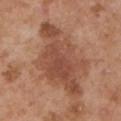Impression:
Imaged during a routine full-body skin examination; the lesion was not biopsied and no histopathology is available.
Background:
Captured under white-light illumination. The patient is a male aged 53 to 57. About 10 mm across. A 15 mm close-up extracted from a 3D total-body photography capture. Located on the arm.